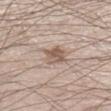Imaged during a routine full-body skin examination; the lesion was not biopsied and no histopathology is available. Cropped from a whole-body photographic skin survey; the tile spans about 15 mm. The patient is a male about 60 years old. Measured at roughly 3 mm in maximum diameter. On the left lower leg.